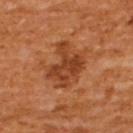follow-up: imaged on a skin check; not biopsied
image: 15 mm crop, total-body photography
patient: male, aged approximately 65
site: the upper back
size: about 5 mm
tile lighting: cross-polarized illumination